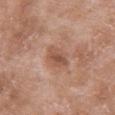Assessment:
The lesion was tiled from a total-body skin photograph and was not biopsied.
Clinical summary:
A close-up tile cropped from a whole-body skin photograph, about 15 mm across. A female subject, about 70 years old. Captured under white-light illumination. The lesion is on the chest.